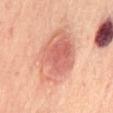notes = imaged on a skin check; not biopsied
acquisition = ~15 mm tile from a whole-body skin photo
subject = male, in their mid- to late 60s
body site = the abdomen